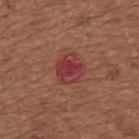Imaged during a routine full-body skin examination; the lesion was not biopsied and no histopathology is available.
Cropped from a total-body skin-imaging series; the visible field is about 15 mm.
The lesion is on the upper back.
The patient is a female roughly 65 years of age.